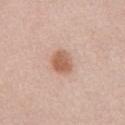The lesion was photographed on a routine skin check and not biopsied; there is no pathology result. The lesion is located on the abdomen. Longest diameter approximately 3 mm. Cropped from a whole-body photographic skin survey; the tile spans about 15 mm. Imaged with white-light lighting. The patient is a male aged around 55.